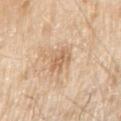{
  "biopsy_status": "not biopsied; imaged during a skin examination",
  "image": {
    "source": "total-body photography crop",
    "field_of_view_mm": 15
  },
  "lighting": "white-light",
  "lesion_size": {
    "long_diameter_mm_approx": 3.0
  },
  "site": "right upper arm",
  "patient": {
    "sex": "male",
    "age_approx": 80
  },
  "automated_metrics": {
    "area_mm2_approx": 4.5,
    "eccentricity": 0.65,
    "shape_asymmetry": 0.25,
    "cielab_L": 64,
    "cielab_a": 19,
    "cielab_b": 35,
    "vs_skin_darker_L": 9.0,
    "vs_skin_contrast_norm": 6.0,
    "border_irregularity_0_10": 3.5,
    "color_variation_0_10": 3.0,
    "peripheral_color_asymmetry": 1.0,
    "nevus_likeness_0_100": 0,
    "lesion_detection_confidence_0_100": 100
  }
}